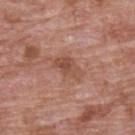Q: Was this lesion biopsied?
A: imaged on a skin check; not biopsied
Q: What kind of image is this?
A: ~15 mm crop, total-body skin-cancer survey
Q: What is the lesion's diameter?
A: ≈4 mm
Q: Who is the patient?
A: male, in their 70s
Q: Lesion location?
A: the upper back
Q: How was the tile lit?
A: white-light illumination